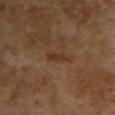– lighting · cross-polarized illumination
– acquisition · 15 mm crop, total-body photography
– diameter · ≈3.5 mm
– patient · female, aged 58–62
– location · the upper back